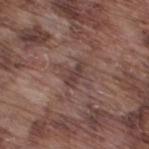Part of a total-body skin-imaging series; this lesion was reviewed on a skin check and was not flagged for biopsy.
The lesion is located on the right thigh.
A male subject in their mid- to late 70s.
Cropped from a whole-body photographic skin survey; the tile spans about 15 mm.
The total-body-photography lesion software estimated a footprint of about 4.5 mm², an eccentricity of roughly 0.85, and two-axis asymmetry of about 0.35. The software also gave a border-irregularity rating of about 3.5/10 and peripheral color asymmetry of about 0.5. It also reported an automated nevus-likeness rating near 0 out of 100 and a detector confidence of about 95 out of 100 that the crop contains a lesion.